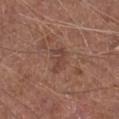Notes:
* biopsy status — no biopsy performed (imaged during a skin exam)
* lesion diameter — about 3 mm
* image source — ~15 mm crop, total-body skin-cancer survey
* patient — male, aged around 75
* body site — the leg